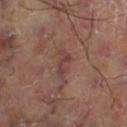Assessment: The lesion was tiled from a total-body skin photograph and was not biopsied. Acquisition and patient details: A 15 mm crop from a total-body photograph taken for skin-cancer surveillance. Measured at roughly 3.5 mm in maximum diameter. The lesion is on the right thigh. Captured under cross-polarized illumination.